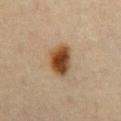Q: Is there a histopathology result?
A: imaged on a skin check; not biopsied
Q: What is the lesion's diameter?
A: ≈4.5 mm
Q: Illumination type?
A: cross-polarized illumination
Q: What is the imaging modality?
A: ~15 mm crop, total-body skin-cancer survey
Q: Lesion location?
A: the abdomen
Q: Automated lesion metrics?
A: an average lesion color of about L≈39 a*≈17 b*≈31 (CIELAB), about 15 CIELAB-L* units darker than the surrounding skin, and a lesion-to-skin contrast of about 12.5 (normalized; higher = more distinct); a detector confidence of about 100 out of 100 that the crop contains a lesion
Q: Patient demographics?
A: female, in their mid- to late 60s